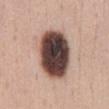Assessment: This lesion was catalogued during total-body skin photography and was not selected for biopsy. Background: Automated tile analysis of the lesion measured a lesion area of about 26 mm², a shape eccentricity near 0.75, and a shape-asymmetry score of about 0.05 (0 = symmetric). It also reported a classifier nevus-likeness of about 20/100 and a detector confidence of about 100 out of 100 that the crop contains a lesion. The lesion is located on the abdomen. A region of skin cropped from a whole-body photographic capture, roughly 15 mm wide. The tile uses white-light illumination. A female subject aged 43 to 47.